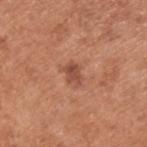Findings:
- notes: catalogued during a skin exam; not biopsied
- body site: the upper back
- lighting: white-light illumination
- patient: male, aged around 55
- lesion size: ~2.5 mm (longest diameter)
- image source: 15 mm crop, total-body photography